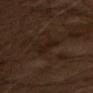Imaged with cross-polarized lighting. A region of skin cropped from a whole-body photographic capture, roughly 15 mm wide. The patient is a male roughly 65 years of age.